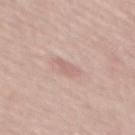Imaged during a routine full-body skin examination; the lesion was not biopsied and no histopathology is available. Approximately 2.5 mm at its widest. A 15 mm crop from a total-body photograph taken for skin-cancer surveillance. Captured under white-light illumination. A female subject, roughly 65 years of age. Automated tile analysis of the lesion measured a nevus-likeness score of about 0/100. On the back.